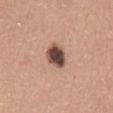Impression: Part of a total-body skin-imaging series; this lesion was reviewed on a skin check and was not flagged for biopsy. Clinical summary: A 15 mm close-up tile from a total-body photography series done for melanoma screening. A female subject, aged 33–37. Automated tile analysis of the lesion measured an eccentricity of roughly 0.75. The analysis additionally found a classifier nevus-likeness of about 70/100. This is a white-light tile. The lesion is on the abdomen. The recorded lesion diameter is about 3.5 mm.